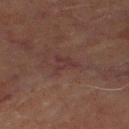Notes:
- follow-up — total-body-photography surveillance lesion; no biopsy
- automated lesion analysis — an average lesion color of about L≈27 a*≈16 b*≈16 (CIELAB), a lesion–skin lightness drop of about 4, and a normalized lesion–skin contrast near 5.5; an automated nevus-likeness rating near 0 out of 100
- patient — male, roughly 70 years of age
- site — the left thigh
- image — ~15 mm crop, total-body skin-cancer survey
- illumination — cross-polarized